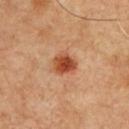  biopsy_status: not biopsied; imaged during a skin examination
  lesion_size:
    long_diameter_mm_approx: 3.0
  patient:
    sex: male
    age_approx: 50
  image:
    source: total-body photography crop
    field_of_view_mm: 15
  site: chest
  automated_metrics:
    eccentricity: 0.45
    shape_asymmetry: 0.25
    cielab_L: 49
    cielab_a: 29
    cielab_b: 37
    vs_skin_contrast_norm: 10.0
    border_irregularity_0_10: 2.0
    color_variation_0_10: 5.5
    peripheral_color_asymmetry: 1.5
    nevus_likeness_0_100: 100
    lesion_detection_confidence_0_100: 100